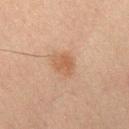Clinical impression: Captured during whole-body skin photography for melanoma surveillance; the lesion was not biopsied. Context: An algorithmic analysis of the crop reported a lesion color around L≈47 a*≈18 b*≈28 in CIELAB, about 7 CIELAB-L* units darker than the surrounding skin, and a normalized lesion–skin contrast near 6. The analysis additionally found a nevus-likeness score of about 50/100 and lesion-presence confidence of about 100/100. A male subject, in their mid-60s. On the leg. A 15 mm crop from a total-body photograph taken for skin-cancer surveillance. This is a cross-polarized tile. Longest diameter approximately 2.5 mm.